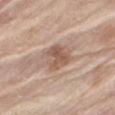Captured during whole-body skin photography for melanoma surveillance; the lesion was not biopsied. The tile uses white-light illumination. The patient is a male aged 78–82. The lesion's longest dimension is about 3.5 mm. A region of skin cropped from a whole-body photographic capture, roughly 15 mm wide. The lesion is on the leg.